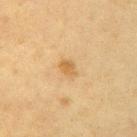Assessment: The lesion was tiled from a total-body skin photograph and was not biopsied. Clinical summary: Approximately 2.5 mm at its widest. A female subject roughly 55 years of age. From the right upper arm. Imaged with cross-polarized lighting. A 15 mm close-up tile from a total-body photography series done for melanoma screening.